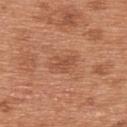No biopsy was performed on this lesion — it was imaged during a full skin examination and was not determined to be concerning.
Automated image analysis of the tile measured roughly 8 lightness units darker than nearby skin and a normalized border contrast of about 5.5. The software also gave a border-irregularity index near 4/10 and radial color variation of about 0.5. The analysis additionally found a nevus-likeness score of about 5/100 and a detector confidence of about 100 out of 100 that the crop contains a lesion.
Cropped from a whole-body photographic skin survey; the tile spans about 15 mm.
Imaged with white-light lighting.
The lesion is located on the upper back.
The lesion's longest dimension is about 3 mm.
A male subject, aged approximately 65.